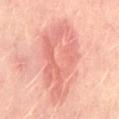biopsy_status: not biopsied; imaged during a skin examination
patient:
  sex: female
  age_approx: 50
automated_metrics:
  area_mm2_approx: 34.0
  eccentricity: 0.9
  shape_asymmetry: 0.3
  cielab_L: 66
  cielab_a: 29
  cielab_b: 29
  vs_skin_darker_L: 10.0
  vs_skin_contrast_norm: 6.0
  border_irregularity_0_10: 5.5
  color_variation_0_10: 4.5
  peripheral_color_asymmetry: 1.5
  nevus_likeness_0_100: 5
  lesion_detection_confidence_0_100: 100
site: front of the torso
image:
  source: total-body photography crop
  field_of_view_mm: 15